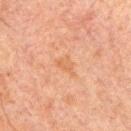Impression: Captured during whole-body skin photography for melanoma surveillance; the lesion was not biopsied. Image and clinical context: Imaged with cross-polarized lighting. A male patient about 60 years old. A roughly 15 mm field-of-view crop from a total-body skin photograph. The lesion's longest dimension is about 3 mm. The lesion is located on the arm.